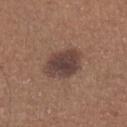<lesion>
<image>
  <source>total-body photography crop</source>
  <field_of_view_mm>15</field_of_view_mm>
</image>
<site>left lower leg</site>
<patient>
  <sex>male</sex>
  <age_approx>65</age_approx>
</patient>
</lesion>